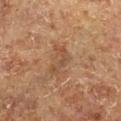The lesion was photographed on a routine skin check and not biopsied; there is no pathology result.
A close-up tile cropped from a whole-body skin photograph, about 15 mm across.
The tile uses cross-polarized illumination.
A male patient, about 75 years old.
An algorithmic analysis of the crop reported a shape eccentricity near 0.85 and a shape-asymmetry score of about 0.65 (0 = symmetric). And it measured a lesion–skin lightness drop of about 6 and a lesion-to-skin contrast of about 5.5 (normalized; higher = more distinct).
About 3 mm across.
On the right lower leg.